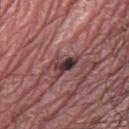* automated metrics · a lesion area of about 5.5 mm², an eccentricity of roughly 0.8, and a shape-asymmetry score of about 0.45 (0 = symmetric); a mean CIELAB color near L≈37 a*≈20 b*≈17, about 12 CIELAB-L* units darker than the surrounding skin, and a lesion-to-skin contrast of about 10 (normalized; higher = more distinct); a within-lesion color-variation index near 9.5/10 and a peripheral color-asymmetry measure near 4.5
* site · the right lower leg
* tile lighting · white-light
* imaging modality · 15 mm crop, total-body photography
* patient · male, about 75 years old